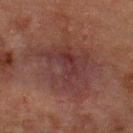Notes:
– biopsy status · total-body-photography surveillance lesion; no biopsy
– acquisition · 15 mm crop, total-body photography
– lighting · cross-polarized illumination
– automated lesion analysis · a lesion area of about 33 mm², a shape eccentricity near 0.6, and a shape-asymmetry score of about 0.4 (0 = symmetric); an average lesion color of about L≈28 a*≈17 b*≈17 (CIELAB), roughly 6 lightness units darker than nearby skin, and a normalized lesion–skin contrast near 7; a lesion-detection confidence of about 75/100
– lesion size · ≈8 mm
– site · the back
– subject · male, aged 48 to 52